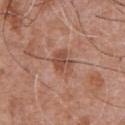No biopsy was performed on this lesion — it was imaged during a full skin examination and was not determined to be concerning.
This image is a 15 mm lesion crop taken from a total-body photograph.
The lesion is on the chest.
Automated image analysis of the tile measured a lesion area of about 6 mm² and two-axis asymmetry of about 0.3. The software also gave a border-irregularity index near 3/10, internal color variation of about 3.5 on a 0–10 scale, and radial color variation of about 1. The analysis additionally found an automated nevus-likeness rating near 0 out of 100 and a lesion-detection confidence of about 100/100.
The subject is a male roughly 75 years of age.
Captured under white-light illumination.